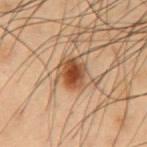Clinical impression:
Imaged during a routine full-body skin examination; the lesion was not biopsied and no histopathology is available.
Image and clinical context:
The lesion-visualizer software estimated an outline eccentricity of about 0.65 (0 = round, 1 = elongated). The software also gave a nevus-likeness score of about 100/100 and a lesion-detection confidence of about 100/100. Captured under cross-polarized illumination. Cropped from a total-body skin-imaging series; the visible field is about 15 mm. The lesion is located on the front of the torso. A male subject, aged 53 to 57. The recorded lesion diameter is about 3.5 mm.